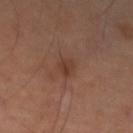The lesion was photographed on a routine skin check and not biopsied; there is no pathology result. A lesion tile, about 15 mm wide, cut from a 3D total-body photograph. A female patient, in their 60s. The lesion is on the right leg. The total-body-photography lesion software estimated an outline eccentricity of about 0.55 (0 = round, 1 = elongated) and two-axis asymmetry of about 0.25. It also reported a mean CIELAB color near L≈39 a*≈21 b*≈27, roughly 6 lightness units darker than nearby skin, and a normalized lesion–skin contrast near 5.5. And it measured a border-irregularity index near 2.5/10, a color-variation rating of about 3/10, and a peripheral color-asymmetry measure near 1. The lesion's longest dimension is about 2.5 mm. Imaged with cross-polarized lighting.